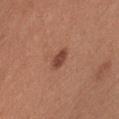Assessment: This lesion was catalogued during total-body skin photography and was not selected for biopsy. Clinical summary: Imaged with white-light lighting. A roughly 15 mm field-of-view crop from a total-body skin photograph. A male subject aged approximately 35. The lesion's longest dimension is about 3 mm. The lesion is on the chest.